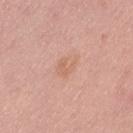Assessment:
The lesion was tiled from a total-body skin photograph and was not biopsied.
Context:
A male patient roughly 40 years of age. A lesion tile, about 15 mm wide, cut from a 3D total-body photograph. On the lower back. The total-body-photography lesion software estimated a border-irregularity index near 3/10, internal color variation of about 2 on a 0–10 scale, and radial color variation of about 1. The analysis additionally found a detector confidence of about 100 out of 100 that the crop contains a lesion. Imaged with white-light lighting. Longest diameter approximately 3 mm.